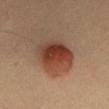Part of a total-body skin-imaging series; this lesion was reviewed on a skin check and was not flagged for biopsy.
The subject is a male aged approximately 40.
Automated image analysis of the tile measured a footprint of about 19 mm², an outline eccentricity of about 0.25 (0 = round, 1 = elongated), and a shape-asymmetry score of about 0.1 (0 = symmetric). It also reported an average lesion color of about L≈42 a*≈23 b*≈29 (CIELAB) and about 12 CIELAB-L* units darker than the surrounding skin. It also reported a color-variation rating of about 9/10 and peripheral color asymmetry of about 2.5. And it measured a detector confidence of about 100 out of 100 that the crop contains a lesion.
On the abdomen.
Approximately 5 mm at its widest.
This is a cross-polarized tile.
A lesion tile, about 15 mm wide, cut from a 3D total-body photograph.